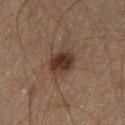biopsy status — catalogued during a skin exam; not biopsied | subject — male, aged around 60 | tile lighting — cross-polarized illumination | image-analysis metrics — a footprint of about 7 mm², a shape eccentricity near 0.45, and a symmetry-axis asymmetry near 0.15; a lesion color around L≈24 a*≈14 b*≈20 in CIELAB and a normalized lesion–skin contrast near 10.5; a border-irregularity rating of about 1.5/10, a color-variation rating of about 3/10, and a peripheral color-asymmetry measure near 1; lesion-presence confidence of about 100/100 | location — the left thigh | image — 15 mm crop, total-body photography | lesion size — ~3 mm (longest diameter).